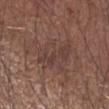This lesion was catalogued during total-body skin photography and was not selected for biopsy. The patient is a male approximately 65 years of age. The recorded lesion diameter is about 8 mm. A 15 mm close-up extracted from a 3D total-body photography capture. The lesion is located on the right forearm.